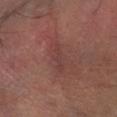biopsy status — catalogued during a skin exam; not biopsied | body site — the right lower leg | image-analysis metrics — a mean CIELAB color near L≈38 a*≈22 b*≈21, about 6 CIELAB-L* units darker than the surrounding skin, and a lesion-to-skin contrast of about 5 (normalized; higher = more distinct); border irregularity of about 6.5 on a 0–10 scale, internal color variation of about 0 on a 0–10 scale, and a peripheral color-asymmetry measure near 0; a classifier nevus-likeness of about 0/100 and a detector confidence of about 75 out of 100 that the crop contains a lesion | illumination — cross-polarized illumination | lesion diameter — ~4 mm (longest diameter) | subject — aged 63–67 | image source — ~15 mm tile from a whole-body skin photo.